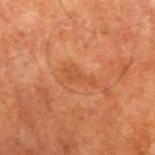notes: total-body-photography surveillance lesion; no biopsy | image: total-body-photography crop, ~15 mm field of view | subject: male, aged approximately 75 | body site: the right upper arm.